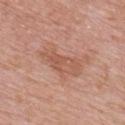biopsy status: catalogued during a skin exam; not biopsied | subject: male, aged 73–77 | location: the mid back | image source: 15 mm crop, total-body photography | lesion size: ~5.5 mm (longest diameter).